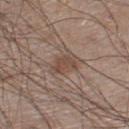Q: Was a biopsy performed?
A: catalogued during a skin exam; not biopsied
Q: What is the lesion's diameter?
A: ~4 mm (longest diameter)
Q: Automated lesion metrics?
A: a footprint of about 7 mm² and a shape-asymmetry score of about 0.25 (0 = symmetric); about 8 CIELAB-L* units darker than the surrounding skin and a normalized border contrast of about 6; a border-irregularity rating of about 3/10 and a peripheral color-asymmetry measure near 1
Q: What lighting was used for the tile?
A: white-light illumination
Q: What is the anatomic site?
A: the right lower leg
Q: Patient demographics?
A: male, aged around 60
Q: What kind of image is this?
A: ~15 mm tile from a whole-body skin photo